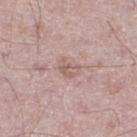body site: the leg
lesion diameter: ~2.5 mm (longest diameter)
acquisition: ~15 mm crop, total-body skin-cancer survey
lighting: white-light
patient: male, in their mid-70s